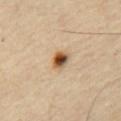The lesion was photographed on a routine skin check and not biopsied; there is no pathology result. The subject is a male approximately 65 years of age. Captured under cross-polarized illumination. This image is a 15 mm lesion crop taken from a total-body photograph. On the chest.